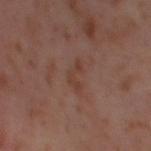  biopsy_status: not biopsied; imaged during a skin examination
  lesion_size:
    long_diameter_mm_approx: 3.5
  lighting: cross-polarized
  automated_metrics:
    area_mm2_approx: 3.5
    eccentricity: 0.9
    shape_asymmetry: 0.7
    cielab_L: 40
    cielab_a: 20
    cielab_b: 26
    vs_skin_contrast_norm: 6.0
    border_irregularity_0_10: 7.5
    nevus_likeness_0_100: 0
    lesion_detection_confidence_0_100: 100
  image:
    source: total-body photography crop
    field_of_view_mm: 15
  site: left thigh
  patient:
    sex: female
    age_approx: 55